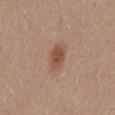{
  "biopsy_status": "not biopsied; imaged during a skin examination"
}